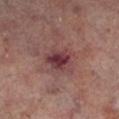{
  "biopsy_status": "not biopsied; imaged during a skin examination",
  "patient": {
    "sex": "male",
    "age_approx": 65
  },
  "site": "left lower leg",
  "image": {
    "source": "total-body photography crop",
    "field_of_view_mm": 15
  },
  "lesion_size": {
    "long_diameter_mm_approx": 3.5
  }
}